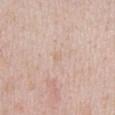Located on the chest. The tile uses white-light illumination. An algorithmic analysis of the crop reported an area of roughly 1.5 mm², an outline eccentricity of about 0.75 (0 = round, 1 = elongated), and a symmetry-axis asymmetry near 0.25. The analysis additionally found a border-irregularity rating of about 2/10, internal color variation of about 0 on a 0–10 scale, and peripheral color asymmetry of about 0. It also reported an automated nevus-likeness rating near 0 out of 100 and lesion-presence confidence of about 100/100. A male patient aged approximately 40. Measured at roughly 1.5 mm in maximum diameter. A close-up tile cropped from a whole-body skin photograph, about 15 mm across.